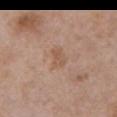biopsy_status: not biopsied; imaged during a skin examination
lesion_size:
  long_diameter_mm_approx: 2.5
image:
  source: total-body photography crop
  field_of_view_mm: 15
site: chest
automated_metrics:
  cielab_L: 55
  cielab_a: 19
  cielab_b: 30
  vs_skin_darker_L: 6.0
patient:
  sex: female
  age_approx: 75
lighting: white-light